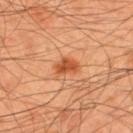Clinical summary:
A male subject aged approximately 45. A 15 mm close-up tile from a total-body photography series done for melanoma screening. Measured at roughly 3 mm in maximum diameter. Captured under cross-polarized illumination. The lesion is on the upper back.